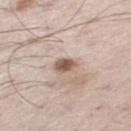Part of a total-body skin-imaging series; this lesion was reviewed on a skin check and was not flagged for biopsy. Imaged with white-light lighting. On the leg. The lesion-visualizer software estimated an area of roughly 4 mm² and a shape eccentricity near 0.7. And it measured a lesion color around L≈56 a*≈17 b*≈25 in CIELAB, a lesion–skin lightness drop of about 15, and a normalized lesion–skin contrast near 9.5. About 2.5 mm across. A roughly 15 mm field-of-view crop from a total-body skin photograph. The patient is a male approximately 55 years of age.